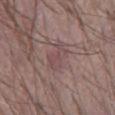Imaged with white-light lighting. The lesion's longest dimension is about 4 mm. Cropped from a total-body skin-imaging series; the visible field is about 15 mm. A male patient in their mid- to late 50s. An algorithmic analysis of the crop reported a footprint of about 6 mm², an eccentricity of roughly 0.85, and two-axis asymmetry of about 0.45.